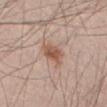Clinical summary: The patient is a male about 35 years old. This is a white-light tile. The recorded lesion diameter is about 3.5 mm. A roughly 15 mm field-of-view crop from a total-body skin photograph. Located on the lower back.